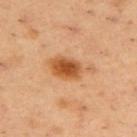Recorded during total-body skin imaging; not selected for excision or biopsy. A male subject, approximately 55 years of age. A close-up tile cropped from a whole-body skin photograph, about 15 mm across. This is a cross-polarized tile. The lesion is on the back. The recorded lesion diameter is about 5.5 mm.